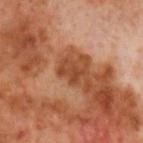A male subject, about 60 years old. Longest diameter approximately 3 mm. Cropped from a whole-body photographic skin survey; the tile spans about 15 mm. An algorithmic analysis of the crop reported an average lesion color of about L≈44 a*≈25 b*≈34 (CIELAB), about 9 CIELAB-L* units darker than the surrounding skin, and a normalized border contrast of about 7.5. It also reported a color-variation rating of about 3.5/10 and peripheral color asymmetry of about 1.5. It also reported a lesion-detection confidence of about 100/100. On the head or neck. The tile uses cross-polarized illumination.